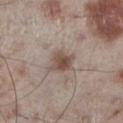Impression: Captured during whole-body skin photography for melanoma surveillance; the lesion was not biopsied. Clinical summary: The tile uses white-light illumination. Automated tile analysis of the lesion measured an area of roughly 6.5 mm² and a shape-asymmetry score of about 0.25 (0 = symmetric). The software also gave a mean CIELAB color near L≈49 a*≈15 b*≈22, about 11 CIELAB-L* units darker than the surrounding skin, and a normalized border contrast of about 8. The lesion is on the leg. A 15 mm close-up tile from a total-body photography series done for melanoma screening. A male subject, in their 60s. Longest diameter approximately 3.5 mm.